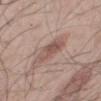Clinical impression: Imaged during a routine full-body skin examination; the lesion was not biopsied and no histopathology is available. Acquisition and patient details: A 15 mm close-up tile from a total-body photography series done for melanoma screening. The subject is a male roughly 55 years of age. The tile uses white-light illumination. On the back.